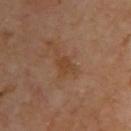Q: What is the imaging modality?
A: ~15 mm tile from a whole-body skin photo
Q: What are the patient's age and sex?
A: male, approximately 65 years of age
Q: What lighting was used for the tile?
A: cross-polarized illumination
Q: Automated lesion metrics?
A: a border-irregularity rating of about 3/10 and a color-variation rating of about 1.5/10; a nevus-likeness score of about 0/100 and a detector confidence of about 100 out of 100 that the crop contains a lesion
Q: Where on the body is the lesion?
A: the upper back
Q: How large is the lesion?
A: ~2.5 mm (longest diameter)